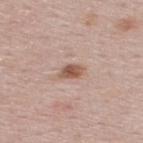This lesion was catalogued during total-body skin photography and was not selected for biopsy.
A region of skin cropped from a whole-body photographic capture, roughly 15 mm wide.
Located on the upper back.
The patient is a male about 60 years old.